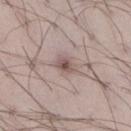biopsy status: imaged on a skin check; not biopsied
automated lesion analysis: a lesion color around L≈53 a*≈17 b*≈19 in CIELAB; an automated nevus-likeness rating near 5 out of 100 and a lesion-detection confidence of about 100/100
illumination: white-light illumination
patient: male, aged 38–42
imaging modality: 15 mm crop, total-body photography
lesion diameter: ≈2.5 mm
body site: the right thigh